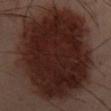Clinical impression:
Recorded during total-body skin imaging; not selected for excision or biopsy.
Acquisition and patient details:
A 15 mm close-up tile from a total-body photography series done for melanoma screening. A male patient aged approximately 50. Imaged with cross-polarized lighting. On the mid back.